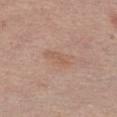Clinical impression:
Recorded during total-body skin imaging; not selected for excision or biopsy.
Context:
The recorded lesion diameter is about 3.5 mm. A 15 mm close-up extracted from a 3D total-body photography capture. Located on the chest. An algorithmic analysis of the crop reported a lesion area of about 4.5 mm², an eccentricity of roughly 0.9, and a symmetry-axis asymmetry near 0.25. It also reported a mean CIELAB color near L≈57 a*≈19 b*≈29 and a normalized border contrast of about 5. The subject is a female in their mid-60s.